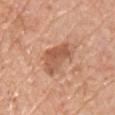{"biopsy_status": "not biopsied; imaged during a skin examination", "patient": {"sex": "male", "age_approx": 60}, "lesion_size": {"long_diameter_mm_approx": 5.0}, "lighting": "white-light", "site": "chest", "image": {"source": "total-body photography crop", "field_of_view_mm": 15}}